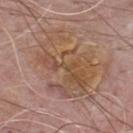Part of a total-body skin-imaging series; this lesion was reviewed on a skin check and was not flagged for biopsy.
The subject is a male aged 73–77.
This is a white-light tile.
Cropped from a whole-body photographic skin survey; the tile spans about 15 mm.
Approximately 8.5 mm at its widest.
Located on the chest.